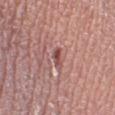  biopsy_status: not biopsied; imaged during a skin examination
  lesion_size:
    long_diameter_mm_approx: 2.5
  image:
    source: total-body photography crop
    field_of_view_mm: 15
  patient:
    sex: female
    age_approx: 40
  site: right lower leg
  lighting: white-light
  automated_metrics:
    area_mm2_approx: 2.5
    shape_asymmetry: 0.3
    border_irregularity_0_10: 3.0
    peripheral_color_asymmetry: 0.0